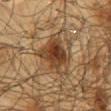The lesion was tiled from a total-body skin photograph and was not biopsied. Measured at roughly 5.5 mm in maximum diameter. This is a cross-polarized tile. This image is a 15 mm lesion crop taken from a total-body photograph. The total-body-photography lesion software estimated a lesion color around L≈39 a*≈17 b*≈31 in CIELAB and a normalized lesion–skin contrast near 10. The software also gave a border-irregularity index near 4/10, internal color variation of about 7 on a 0–10 scale, and radial color variation of about 2. A male subject in their mid-60s. The lesion is located on the mid back.